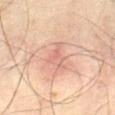The lesion was photographed on a routine skin check and not biopsied; there is no pathology result.
On the abdomen.
Automated image analysis of the tile measured a lesion–skin lightness drop of about 7.
A lesion tile, about 15 mm wide, cut from a 3D total-body photograph.
A male subject aged 63–67.
Longest diameter approximately 3.5 mm.